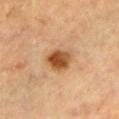notes — no biopsy performed (imaged during a skin exam)
automated metrics — an eccentricity of roughly 0.45 and a shape-asymmetry score of about 0.2 (0 = symmetric); border irregularity of about 1.5 on a 0–10 scale, a within-lesion color-variation index near 6/10, and radial color variation of about 2; an automated nevus-likeness rating near 100 out of 100
body site — the right upper arm
image — ~15 mm tile from a whole-body skin photo
lighting — cross-polarized illumination
patient — male, in their mid-60s